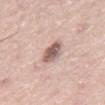{"biopsy_status": "not biopsied; imaged during a skin examination", "automated_metrics": {"area_mm2_approx": 6.5, "shape_asymmetry": 0.15, "border_irregularity_0_10": 1.5, "color_variation_0_10": 4.5, "peripheral_color_asymmetry": 1.5, "nevus_likeness_0_100": 60, "lesion_detection_confidence_0_100": 100}, "site": "chest", "lighting": "white-light", "patient": {"sex": "male", "age_approx": 75}, "image": {"source": "total-body photography crop", "field_of_view_mm": 15}}